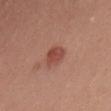| feature | finding |
|---|---|
| follow-up | total-body-photography surveillance lesion; no biopsy |
| lesion diameter | ≈3 mm |
| image source | 15 mm crop, total-body photography |
| site | the back |
| subject | male, aged 58 to 62 |
| tile lighting | white-light illumination |